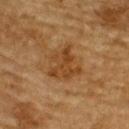Findings:
* workup · total-body-photography surveillance lesion; no biopsy
* subject · male, aged 83 or older
* imaging modality · total-body-photography crop, ~15 mm field of view
* lesion diameter · ≈4.5 mm
* location · the upper back
* illumination · cross-polarized illumination